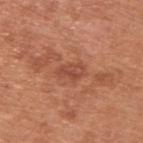Q: Is there a histopathology result?
A: no biopsy performed (imaged during a skin exam)
Q: What lighting was used for the tile?
A: white-light
Q: Patient demographics?
A: male, in their mid- to late 60s
Q: What kind of image is this?
A: 15 mm crop, total-body photography
Q: Lesion size?
A: about 3 mm
Q: Where on the body is the lesion?
A: the back